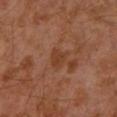workup — imaged on a skin check; not biopsied | location — the left upper arm | illumination — cross-polarized illumination | subject — male, roughly 30 years of age | image source — 15 mm crop, total-body photography | automated metrics — border irregularity of about 3 on a 0–10 scale; a nevus-likeness score of about 0/100 and a lesion-detection confidence of about 100/100.